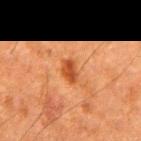No biopsy was performed on this lesion — it was imaged during a full skin examination and was not determined to be concerning. Located on the arm. A lesion tile, about 15 mm wide, cut from a 3D total-body photograph. The total-body-photography lesion software estimated a lesion area of about 4 mm² and a shape-asymmetry score of about 0.3 (0 = symmetric). It also reported a mean CIELAB color near L≈40 a*≈26 b*≈36, about 10 CIELAB-L* units darker than the surrounding skin, and a normalized lesion–skin contrast near 8.5. And it measured a border-irregularity rating of about 2.5/10, a color-variation rating of about 2.5/10, and peripheral color asymmetry of about 1. The lesion's longest dimension is about 2.5 mm. A male subject about 60 years old. This is a cross-polarized tile.